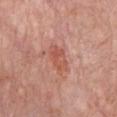Q: Is there a histopathology result?
A: catalogued during a skin exam; not biopsied
Q: What are the patient's age and sex?
A: male, roughly 80 years of age
Q: What is the lesion's diameter?
A: ≈3.5 mm
Q: What kind of image is this?
A: 15 mm crop, total-body photography
Q: How was the tile lit?
A: white-light
Q: What is the anatomic site?
A: the front of the torso